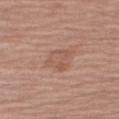A male patient, aged 58–62.
The lesion is located on the right upper arm.
A region of skin cropped from a whole-body photographic capture, roughly 15 mm wide.